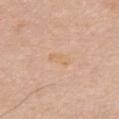Findings:
* notes · imaged on a skin check; not biopsied
* size · ≈3 mm
* illumination · white-light illumination
* imaging modality · 15 mm crop, total-body photography
* subject · male, approximately 70 years of age
* body site · the chest